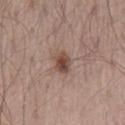biopsy_status: not biopsied; imaged during a skin examination
lighting: white-light
image:
  source: total-body photography crop
  field_of_view_mm: 15
lesion_size:
  long_diameter_mm_approx: 2.5
patient:
  sex: male
  age_approx: 65
site: back